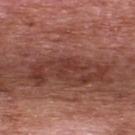The lesion was photographed on a routine skin check and not biopsied; there is no pathology result. A 15 mm close-up tile from a total-body photography series done for melanoma screening. Approximately 2.5 mm at its widest. A male patient, aged approximately 70. The tile uses white-light illumination. From the upper back.